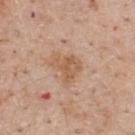biopsy status — no biopsy performed (imaged during a skin exam); patient — male, in their 60s; automated metrics — an area of roughly 6.5 mm², an outline eccentricity of about 0.5 (0 = round, 1 = elongated), and two-axis asymmetry of about 0.5; site — the upper back; imaging modality — ~15 mm tile from a whole-body skin photo; lesion diameter — ~3 mm (longest diameter); tile lighting — white-light illumination.